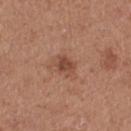biopsy status = catalogued during a skin exam; not biopsied | automated metrics = a detector confidence of about 100 out of 100 that the crop contains a lesion | patient = female, roughly 40 years of age | lighting = white-light | site = the left thigh | lesion size = ≈3 mm | image = 15 mm crop, total-body photography.